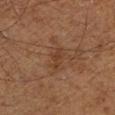{
  "biopsy_status": "not biopsied; imaged during a skin examination",
  "automated_metrics": {
    "cielab_L": 29,
    "cielab_a": 17,
    "cielab_b": 25,
    "vs_skin_darker_L": 5.0,
    "nevus_likeness_0_100": 0
  },
  "lighting": "cross-polarized",
  "site": "left lower leg",
  "image": {
    "source": "total-body photography crop",
    "field_of_view_mm": 15
  },
  "lesion_size": {
    "long_diameter_mm_approx": 3.0
  },
  "patient": {
    "sex": "male",
    "age_approx": 75
  }
}